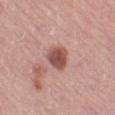<record>
<biopsy_status>not biopsied; imaged during a skin examination</biopsy_status>
<lesion_size>
  <long_diameter_mm_approx>3.5</long_diameter_mm_approx>
</lesion_size>
<patient>
  <sex>female</sex>
  <age_approx>50</age_approx>
</patient>
<site>left thigh</site>
<image>
  <source>total-body photography crop</source>
  <field_of_view_mm>15</field_of_view_mm>
</image>
<lighting>white-light</lighting>
</record>